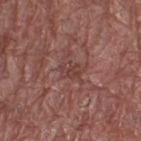notes = no biopsy performed (imaged during a skin exam); size = ~2.5 mm (longest diameter); illumination = white-light illumination; site = the leg; patient = male, roughly 75 years of age; image source = 15 mm crop, total-body photography.